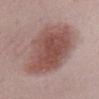Clinical impression: The lesion was photographed on a routine skin check and not biopsied; there is no pathology result. Image and clinical context: Located on the abdomen. The patient is a female aged approximately 40. A region of skin cropped from a whole-body photographic capture, roughly 15 mm wide. Longest diameter approximately 10.5 mm.